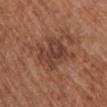No biopsy was performed on this lesion — it was imaged during a full skin examination and was not determined to be concerning. On the upper back. Captured under white-light illumination. A region of skin cropped from a whole-body photographic capture, roughly 15 mm wide. The patient is a male aged 68–72. About 6 mm across.